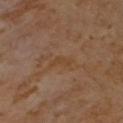<record>
  <image>
    <source>total-body photography crop</source>
    <field_of_view_mm>15</field_of_view_mm>
  </image>
  <site>right upper arm</site>
  <patient>
    <sex>female</sex>
    <age_approx>55</age_approx>
  </patient>
  <lighting>cross-polarized</lighting>
  <lesion_size>
    <long_diameter_mm_approx>2.5</long_diameter_mm_approx>
  </lesion_size>
</record>